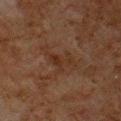follow-up = no biopsy performed (imaged during a skin exam) | diameter = ≈3 mm | patient = male, aged 78 to 82 | image source = 15 mm crop, total-body photography | body site = the chest.